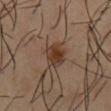Assessment:
The lesion was tiled from a total-body skin photograph and was not biopsied.
Image and clinical context:
Measured at roughly 3.5 mm in maximum diameter. Cropped from a total-body skin-imaging series; the visible field is about 15 mm. A male subject in their mid-50s. On the chest.